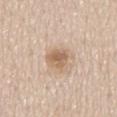Clinical impression: Imaged during a routine full-body skin examination; the lesion was not biopsied and no histopathology is available. Image and clinical context: Automated image analysis of the tile measured an area of roughly 6.5 mm², a shape eccentricity near 0.6, and a symmetry-axis asymmetry near 0.25. It also reported a mean CIELAB color near L≈62 a*≈16 b*≈31, a lesion–skin lightness drop of about 11, and a normalized border contrast of about 7.5. The software also gave a border-irregularity rating of about 2.5/10, internal color variation of about 3.5 on a 0–10 scale, and a peripheral color-asymmetry measure near 1. It also reported a classifier nevus-likeness of about 50/100. A region of skin cropped from a whole-body photographic capture, roughly 15 mm wide. A female subject aged approximately 60. About 3.5 mm across. The lesion is located on the mid back.